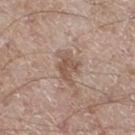Imaged during a routine full-body skin examination; the lesion was not biopsied and no histopathology is available. Measured at roughly 4 mm in maximum diameter. Cropped from a whole-body photographic skin survey; the tile spans about 15 mm. The subject is a male in their mid-60s. The lesion is located on the leg. This is a white-light tile.